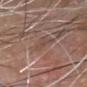biopsy status: imaged on a skin check; not biopsied
tile lighting: white-light
image-analysis metrics: a lesion–skin lightness drop of about 6 and a lesion-to-skin contrast of about 4.5 (normalized; higher = more distinct)
patient: male, aged around 80
lesion size: ~3 mm (longest diameter)
location: the head or neck
imaging modality: ~15 mm tile from a whole-body skin photo